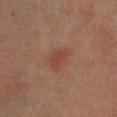Impression:
No biopsy was performed on this lesion — it was imaged during a full skin examination and was not determined to be concerning.
Image and clinical context:
A roughly 15 mm field-of-view crop from a total-body skin photograph. A male patient approximately 70 years of age. Imaged with cross-polarized lighting. Located on the left lower leg.